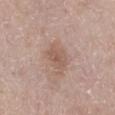– notes — catalogued during a skin exam; not biopsied
– patient — female, aged approximately 65
– lesion size — about 3 mm
– lighting — white-light
– location — the left lower leg
– image source — total-body-photography crop, ~15 mm field of view